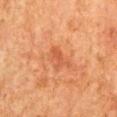Q: Was a biopsy performed?
A: imaged on a skin check; not biopsied
Q: How was this image acquired?
A: total-body-photography crop, ~15 mm field of view
Q: What did automated image analysis measure?
A: a border-irregularity index near 3.5/10 and peripheral color asymmetry of about 1
Q: Patient demographics?
A: male, in their 80s
Q: What is the anatomic site?
A: the chest
Q: How large is the lesion?
A: ~3.5 mm (longest diameter)